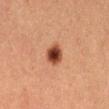notes: catalogued during a skin exam; not biopsied | subject: female, about 40 years old | image source: ~15 mm crop, total-body skin-cancer survey | site: the abdomen | lighting: cross-polarized illumination | diameter: about 2.5 mm.